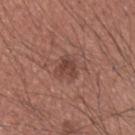The lesion was photographed on a routine skin check and not biopsied; there is no pathology result. The lesion's longest dimension is about 2.5 mm. On the back. An algorithmic analysis of the crop reported a footprint of about 4 mm², a shape eccentricity near 0.4, and a shape-asymmetry score of about 0.4 (0 = symmetric). And it measured a border-irregularity index near 4/10, a within-lesion color-variation index near 3/10, and a peripheral color-asymmetry measure near 1. And it measured a classifier nevus-likeness of about 15/100 and lesion-presence confidence of about 100/100. A male patient, approximately 35 years of age. Cropped from a total-body skin-imaging series; the visible field is about 15 mm.